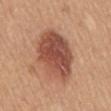Impression: No biopsy was performed on this lesion — it was imaged during a full skin examination and was not determined to be concerning.